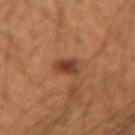{
  "biopsy_status": "not biopsied; imaged during a skin examination",
  "site": "arm",
  "image": {
    "source": "total-body photography crop",
    "field_of_view_mm": 15
  },
  "patient": {
    "sex": "male",
    "age_approx": 20
  }
}